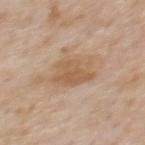biopsy status = total-body-photography surveillance lesion; no biopsy | tile lighting = white-light | site = the back | acquisition = 15 mm crop, total-body photography | diameter = about 5 mm | image-analysis metrics = a mean CIELAB color near L≈58 a*≈17 b*≈33, roughly 8 lightness units darker than nearby skin, and a normalized lesion–skin contrast near 6.5 | patient = male, aged around 60.